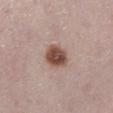Findings:
- notes — total-body-photography surveillance lesion; no biopsy
- patient — male, aged 58–62
- acquisition — ~15 mm crop, total-body skin-cancer survey
- lighting — white-light
- diameter — about 3.5 mm
- TBP lesion metrics — a border-irregularity index near 1.5/10, internal color variation of about 4.5 on a 0–10 scale, and peripheral color asymmetry of about 1.5
- anatomic site — the right lower leg